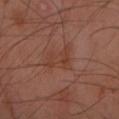No biopsy was performed on this lesion — it was imaged during a full skin examination and was not determined to be concerning. The lesion-visualizer software estimated a footprint of about 8 mm², an outline eccentricity of about 0.7 (0 = round, 1 = elongated), and a symmetry-axis asymmetry near 0.3. A 15 mm close-up extracted from a 3D total-body photography capture. Imaged with cross-polarized lighting. The recorded lesion diameter is about 4 mm. A female subject, aged around 55. The lesion is on the left forearm.